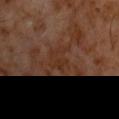| feature | finding |
|---|---|
| anatomic site | the chest |
| image source | ~15 mm tile from a whole-body skin photo |
| subject | male, aged approximately 60 |
| TBP lesion metrics | a lesion area of about 6.5 mm² and a shape eccentricity near 0.55; a mean CIELAB color near L≈29 a*≈18 b*≈26, roughly 4 lightness units darker than nearby skin, and a lesion-to-skin contrast of about 5.5 (normalized; higher = more distinct); internal color variation of about 2.5 on a 0–10 scale and radial color variation of about 1 |
| illumination | cross-polarized illumination |
| diameter | ≈3.5 mm |